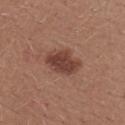biopsy status — imaged on a skin check; not biopsied | tile lighting — white-light | image source — total-body-photography crop, ~15 mm field of view | size — ≈4.5 mm | patient — female, aged 23 to 27 | body site — the upper back.